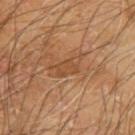follow-up = catalogued during a skin exam; not biopsied
image source = 15 mm crop, total-body photography
location = the left upper arm
tile lighting = cross-polarized
automated metrics = a classifier nevus-likeness of about 0/100 and a lesion-detection confidence of about 95/100
patient = male, aged approximately 65